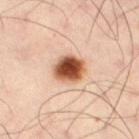<record>
  <biopsy_status>not biopsied; imaged during a skin examination</biopsy_status>
  <lesion_size>
    <long_diameter_mm_approx>4.0</long_diameter_mm_approx>
  </lesion_size>
  <site>lower back</site>
  <image>
    <source>total-body photography crop</source>
    <field_of_view_mm>15</field_of_view_mm>
  </image>
  <patient>
    <sex>male</sex>
    <age_approx>50</age_approx>
  </patient>
</record>